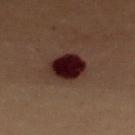The lesion was tiled from a total-body skin photograph and was not biopsied. The subject is a female aged 48–52. Imaged with cross-polarized lighting. Measured at roughly 4.5 mm in maximum diameter. A 15 mm close-up tile from a total-body photography series done for melanoma screening. Located on the chest.